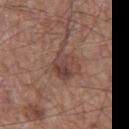Clinical impression: The lesion was photographed on a routine skin check and not biopsied; there is no pathology result. Context: From the left thigh. Captured under white-light illumination. A 15 mm close-up tile from a total-body photography series done for melanoma screening. The patient is a male aged 58 to 62. The recorded lesion diameter is about 4.5 mm. The lesion-visualizer software estimated roughly 8 lightness units darker than nearby skin.